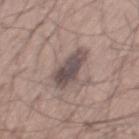Acquisition and patient details: The lesion is on the mid back. A male patient, aged 33 to 37. Cropped from a total-body skin-imaging series; the visible field is about 15 mm. The tile uses white-light illumination. Automated tile analysis of the lesion measured a border-irregularity index near 3.5/10 and a peripheral color-asymmetry measure near 1. About 5.5 mm across.